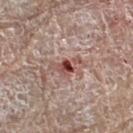notes = no biopsy performed (imaged during a skin exam) | acquisition = ~15 mm tile from a whole-body skin photo | site = the right lower leg | diameter = ≈3.5 mm | subject = female, in their mid- to late 70s.